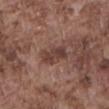Findings:
- workup · total-body-photography surveillance lesion; no biopsy
- image source · total-body-photography crop, ~15 mm field of view
- patient · male, aged 73 to 77
- body site · the front of the torso